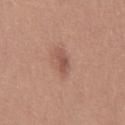<record>
<site>right thigh</site>
<lesion_size>
  <long_diameter_mm_approx>3.0</long_diameter_mm_approx>
</lesion_size>
<automated_metrics>
  <shape_asymmetry>0.3</shape_asymmetry>
  <cielab_L>52</cielab_L>
  <cielab_a>23</cielab_a>
  <cielab_b>26</cielab_b>
  <vs_skin_darker_L>10.0</vs_skin_darker_L>
  <vs_skin_contrast_norm>7.0</vs_skin_contrast_norm>
  <nevus_likeness_0_100>35</nevus_likeness_0_100>
  <lesion_detection_confidence_0_100>100</lesion_detection_confidence_0_100>
</automated_metrics>
<image>
  <source>total-body photography crop</source>
  <field_of_view_mm>15</field_of_view_mm>
</image>
<lighting>white-light</lighting>
<patient>
  <sex>female</sex>
  <age_approx>30</age_approx>
</patient>
</record>